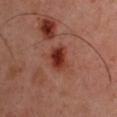Imaged with cross-polarized lighting. Located on the arm. A male patient about 45 years old. A roughly 15 mm field-of-view crop from a total-body skin photograph.